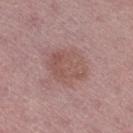- follow-up — catalogued during a skin exam; not biopsied
- lesion diameter — ≈5 mm
- tile lighting — white-light illumination
- site — the right thigh
- patient — female, aged 43–47
- image source — 15 mm crop, total-body photography